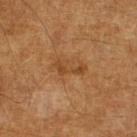subject: male, approximately 65 years of age | diameter: ~4 mm (longest diameter) | TBP lesion metrics: a footprint of about 4.5 mm², an eccentricity of roughly 0.9, and a symmetry-axis asymmetry near 0.5; a border-irregularity rating of about 6.5/10, a within-lesion color-variation index near 1/10, and a peripheral color-asymmetry measure near 0.5; a nevus-likeness score of about 0/100 and a lesion-detection confidence of about 100/100 | image: ~15 mm crop, total-body skin-cancer survey | illumination: cross-polarized illumination.